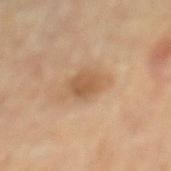Impression:
Part of a total-body skin-imaging series; this lesion was reviewed on a skin check and was not flagged for biopsy.
Image and clinical context:
This is a cross-polarized tile. Cropped from a whole-body photographic skin survey; the tile spans about 15 mm. Longest diameter approximately 4.5 mm. The lesion is located on the mid back. The subject is a male aged 83 or older.